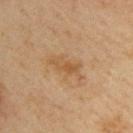biopsy status = catalogued during a skin exam; not biopsied | image = ~15 mm crop, total-body skin-cancer survey | lighting = cross-polarized illumination | site = the upper back | patient = male, roughly 40 years of age.